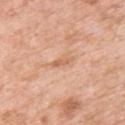Notes:
- workup: total-body-photography surveillance lesion; no biopsy
- subject: female, aged approximately 50
- tile lighting: white-light illumination
- location: the upper back
- size: ~3 mm (longest diameter)
- imaging modality: ~15 mm crop, total-body skin-cancer survey